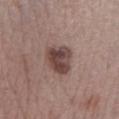{
  "biopsy_status": "not biopsied; imaged during a skin examination",
  "image": {
    "source": "total-body photography crop",
    "field_of_view_mm": 15
  },
  "site": "left lower leg",
  "lighting": "white-light",
  "lesion_size": {
    "long_diameter_mm_approx": 3.5
  },
  "patient": {
    "sex": "male",
    "age_approx": 75
  }
}